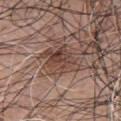lighting: white-light
image:
  source: total-body photography crop
  field_of_view_mm: 15
lesion_size:
  long_diameter_mm_approx: 4.5
site: front of the torso
patient:
  sex: male
  age_approx: 75